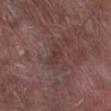Q: Was this lesion biopsied?
A: imaged on a skin check; not biopsied
Q: What did automated image analysis measure?
A: a footprint of about 3.5 mm², a shape eccentricity near 0.8, and two-axis asymmetry of about 0.45; a lesion color around L≈36 a*≈18 b*≈19 in CIELAB; a border-irregularity index near 4.5/10, a color-variation rating of about 2/10, and a peripheral color-asymmetry measure near 0.5; an automated nevus-likeness rating near 0 out of 100
Q: Patient demographics?
A: male, aged approximately 80
Q: What is the anatomic site?
A: the right lower leg
Q: How was this image acquired?
A: ~15 mm tile from a whole-body skin photo
Q: How large is the lesion?
A: ≈3 mm
Q: What lighting was used for the tile?
A: white-light